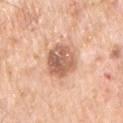Background:
Imaged with white-light lighting. Cropped from a total-body skin-imaging series; the visible field is about 15 mm. Automated image analysis of the tile measured a nevus-likeness score of about 15/100. A male subject, aged around 70. The lesion is located on the chest.